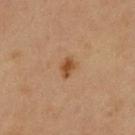Case summary:
- biopsy status · catalogued during a skin exam; not biopsied
- lesion diameter · ~2.5 mm (longest diameter)
- site · the right upper arm
- subject · male, roughly 60 years of age
- acquisition · 15 mm crop, total-body photography